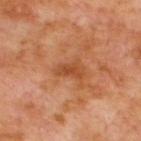subject = male, roughly 70 years of age; location = the upper back; image source = ~15 mm crop, total-body skin-cancer survey.